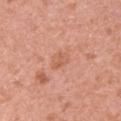Impression:
Captured during whole-body skin photography for melanoma surveillance; the lesion was not biopsied.
Background:
This is a white-light tile. A 15 mm close-up tile from a total-body photography series done for melanoma screening. Located on the right upper arm. Automated tile analysis of the lesion measured an area of roughly 4 mm², an outline eccentricity of about 0.75 (0 = round, 1 = elongated), and a shape-asymmetry score of about 0.25 (0 = symmetric). It also reported an average lesion color of about L≈60 a*≈26 b*≈33 (CIELAB) and a normalized border contrast of about 5. The software also gave internal color variation of about 2 on a 0–10 scale and a peripheral color-asymmetry measure near 0.5. The patient is a female aged approximately 40.